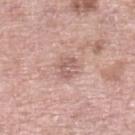Impression: Imaged during a routine full-body skin examination; the lesion was not biopsied and no histopathology is available. Background: The recorded lesion diameter is about 3 mm. A male patient aged 53 to 57. The lesion is on the left lower leg. The total-body-photography lesion software estimated a mean CIELAB color near L≈59 a*≈21 b*≈23, roughly 9 lightness units darker than nearby skin, and a normalized border contrast of about 6. And it measured border irregularity of about 3.5 on a 0–10 scale and a within-lesion color-variation index near 2/10. The analysis additionally found a classifier nevus-likeness of about 0/100 and lesion-presence confidence of about 100/100. Cropped from a whole-body photographic skin survey; the tile spans about 15 mm.